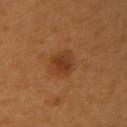Captured during whole-body skin photography for melanoma surveillance; the lesion was not biopsied.
Captured under cross-polarized illumination.
Cropped from a whole-body photographic skin survey; the tile spans about 15 mm.
A female patient, in their mid- to late 40s.
The lesion is located on the arm.